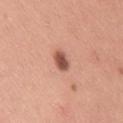Clinical impression: The lesion was photographed on a routine skin check and not biopsied; there is no pathology result. Background: The lesion is on the leg. Cropped from a total-body skin-imaging series; the visible field is about 15 mm. A female subject approximately 30 years of age. This is a white-light tile. Measured at roughly 3 mm in maximum diameter.